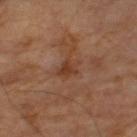image — ~15 mm crop, total-body skin-cancer survey | lighting — cross-polarized illumination | lesion size — ~3 mm (longest diameter) | image-analysis metrics — a nevus-likeness score of about 0/100 | subject — male, roughly 65 years of age.